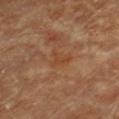biopsy_status: not biopsied; imaged during a skin examination
patient:
  sex: female
  age_approx: 80
site: chest
image:
  source: total-body photography crop
  field_of_view_mm: 15